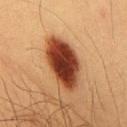The lesion was photographed on a routine skin check and not biopsied; there is no pathology result. A 15 mm crop from a total-body photograph taken for skin-cancer surveillance. From the front of the torso. Imaged with cross-polarized lighting. A male patient about 40 years old. Measured at roughly 7 mm in maximum diameter. The lesion-visualizer software estimated roughly 20 lightness units darker than nearby skin and a normalized border contrast of about 16.5. The analysis additionally found a classifier nevus-likeness of about 100/100.